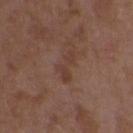Clinical impression: Imaged during a routine full-body skin examination; the lesion was not biopsied and no histopathology is available. Background: The patient is a female approximately 35 years of age. A close-up tile cropped from a whole-body skin photograph, about 15 mm across. Imaged with white-light lighting. On the upper back.